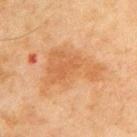follow-up — no biopsy performed (imaged during a skin exam)
site — the upper back
image source — ~15 mm crop, total-body skin-cancer survey
patient — male, aged 43 to 47
automated lesion analysis — an area of roughly 21 mm², a shape eccentricity near 0.8, and two-axis asymmetry of about 0.55; an average lesion color of about L≈50 a*≈20 b*≈35 (CIELAB); a border-irregularity index near 6.5/10 and a peripheral color-asymmetry measure near 1; a classifier nevus-likeness of about 0/100 and lesion-presence confidence of about 100/100
tile lighting — cross-polarized
lesion size — ≈7.5 mm